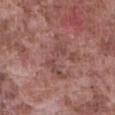No biopsy was performed on this lesion — it was imaged during a full skin examination and was not determined to be concerning. The subject is a male aged 73 to 77. Captured under white-light illumination. An algorithmic analysis of the crop reported a shape eccentricity near 0.9 and two-axis asymmetry of about 0.6. It also reported a mean CIELAB color near L≈47 a*≈22 b*≈22, a lesion–skin lightness drop of about 7, and a normalized lesion–skin contrast near 5.5. It also reported an automated nevus-likeness rating near 0 out of 100 and a detector confidence of about 95 out of 100 that the crop contains a lesion. From the abdomen. The recorded lesion diameter is about 5.5 mm. A roughly 15 mm field-of-view crop from a total-body skin photograph.